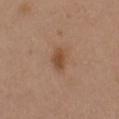notes: imaged on a skin check; not biopsied | subject: female, aged 38 to 42 | site: the right upper arm | image source: ~15 mm crop, total-body skin-cancer survey.